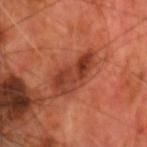Q: Was a biopsy performed?
A: no biopsy performed (imaged during a skin exam)
Q: How large is the lesion?
A: ≈5.5 mm
Q: Where on the body is the lesion?
A: the head or neck
Q: What are the patient's age and sex?
A: male, aged around 70
Q: What kind of image is this?
A: ~15 mm tile from a whole-body skin photo
Q: Illumination type?
A: cross-polarized